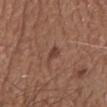Assessment: This lesion was catalogued during total-body skin photography and was not selected for biopsy. Acquisition and patient details: A male patient aged 63–67. The lesion is located on the mid back. Approximately 2.5 mm at its widest. Cropped from a whole-body photographic skin survey; the tile spans about 15 mm. The tile uses white-light illumination.